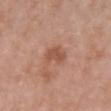Impression: The lesion was photographed on a routine skin check and not biopsied; there is no pathology result. Acquisition and patient details: The lesion is located on the chest. The tile uses white-light illumination. A female subject roughly 65 years of age. A close-up tile cropped from a whole-body skin photograph, about 15 mm across. About 3 mm across.